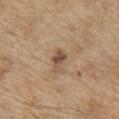Impression:
The lesion was tiled from a total-body skin photograph and was not biopsied.
Context:
Measured at roughly 2.5 mm in maximum diameter. A male subject, aged 68–72. Captured under white-light illumination. Located on the chest. A region of skin cropped from a whole-body photographic capture, roughly 15 mm wide.